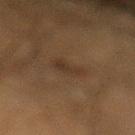Assessment:
The lesion was tiled from a total-body skin photograph and was not biopsied.
Image and clinical context:
The recorded lesion diameter is about 3 mm. A region of skin cropped from a whole-body photographic capture, roughly 15 mm wide. A male subject in their 50s. From the left lower leg. An algorithmic analysis of the crop reported an outline eccentricity of about 0.75 (0 = round, 1 = elongated) and two-axis asymmetry of about 0.35.